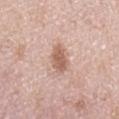workup: total-body-photography surveillance lesion; no biopsy | lighting: white-light | anatomic site: the left upper arm | image: 15 mm crop, total-body photography | patient: female, aged 48–52.